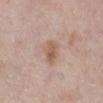biopsy status = total-body-photography surveillance lesion; no biopsy | imaging modality = ~15 mm crop, total-body skin-cancer survey | TBP lesion metrics = a mean CIELAB color near L≈58 a*≈18 b*≈28 and a lesion–skin lightness drop of about 9; an automated nevus-likeness rating near 35 out of 100 and lesion-presence confidence of about 100/100 | lighting = white-light | subject = female, in their mid- to late 60s | size = ≈3 mm | location = the right lower leg.